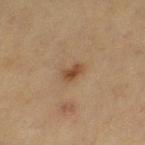biopsy_status: not biopsied; imaged during a skin examination
site: left thigh
image:
  source: total-body photography crop
  field_of_view_mm: 15
patient:
  sex: female
  age_approx: 50
lighting: cross-polarized